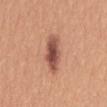Part of a total-body skin-imaging series; this lesion was reviewed on a skin check and was not flagged for biopsy. A female subject aged 38–42. This image is a 15 mm lesion crop taken from a total-body photograph. The lesion is located on the mid back.